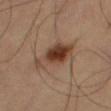The lesion was tiled from a total-body skin photograph and was not biopsied.